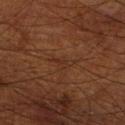biopsy_status: not biopsied; imaged during a skin examination
patient:
  sex: male
  age_approx: 70
image:
  source: total-body photography crop
  field_of_view_mm: 15
lesion_size:
  long_diameter_mm_approx: 2.5
site: right thigh
lighting: cross-polarized